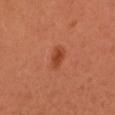The lesion was tiled from a total-body skin photograph and was not biopsied. The patient is a female aged approximately 50. The total-body-photography lesion software estimated a lesion area of about 4.5 mm², a shape eccentricity near 0.8, and a shape-asymmetry score of about 0.2 (0 = symmetric). Cropped from a whole-body photographic skin survey; the tile spans about 15 mm.